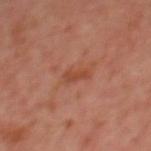Recorded during total-body skin imaging; not selected for excision or biopsy.
The tile uses cross-polarized illumination.
A male subject in their mid- to late 60s.
A region of skin cropped from a whole-body photographic capture, roughly 15 mm wide.
From the upper back.
The lesion-visualizer software estimated a footprint of about 2.5 mm². The analysis additionally found an average lesion color of about L≈44 a*≈26 b*≈32 (CIELAB) and a normalized lesion–skin contrast near 6.5. The analysis additionally found internal color variation of about 0 on a 0–10 scale.